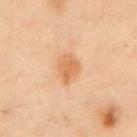Q: Was a biopsy performed?
A: imaged on a skin check; not biopsied
Q: How was this image acquired?
A: ~15 mm crop, total-body skin-cancer survey
Q: Patient demographics?
A: male, in their mid- to late 50s
Q: Where on the body is the lesion?
A: the chest
Q: Illumination type?
A: cross-polarized